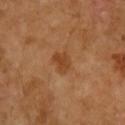biopsy status=imaged on a skin check; not biopsied
lighting=cross-polarized illumination
acquisition=~15 mm tile from a whole-body skin photo
patient=female, aged approximately 70
site=the arm